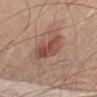biopsy_status: not biopsied; imaged during a skin examination
automated_metrics:
  area_mm2_approx: 9.0
  shape_asymmetry: 0.3
  nevus_likeness_0_100: 55
  lesion_detection_confidence_0_100: 100
site: left upper arm
patient:
  sex: male
  age_approx: 70
lighting: white-light
lesion_size:
  long_diameter_mm_approx: 4.5
image:
  source: total-body photography crop
  field_of_view_mm: 15